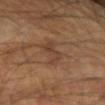diameter: ~4.5 mm (longest diameter)
location: the left forearm
imaging modality: 15 mm crop, total-body photography
subject: male, approximately 65 years of age
automated metrics: an area of roughly 5.5 mm², an outline eccentricity of about 0.9 (0 = round, 1 = elongated), and a shape-asymmetry score of about 0.55 (0 = symmetric)